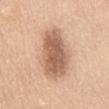Q: Is there a histopathology result?
A: no biopsy performed (imaged during a skin exam)
Q: What are the patient's age and sex?
A: female, in their mid- to late 50s
Q: What lighting was used for the tile?
A: white-light illumination
Q: Where on the body is the lesion?
A: the mid back
Q: What is the imaging modality?
A: 15 mm crop, total-body photography
Q: Lesion size?
A: ~8 mm (longest diameter)